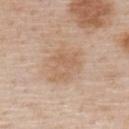* biopsy status — no biopsy performed (imaged during a skin exam)
* automated metrics — a border-irregularity rating of about 6/10, a color-variation rating of about 3/10, and a peripheral color-asymmetry measure near 1
* subject — male, roughly 60 years of age
* image — ~15 mm tile from a whole-body skin photo
* diameter — ≈4 mm
* anatomic site — the upper back
* illumination — white-light illumination